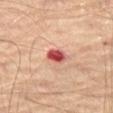| key | value |
|---|---|
| biopsy status | total-body-photography surveillance lesion; no biopsy |
| size | ≈2.5 mm |
| body site | the left thigh |
| image | ~15 mm tile from a whole-body skin photo |
| subject | male, aged around 65 |
| illumination | cross-polarized illumination |